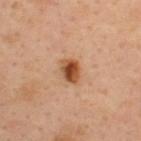<case>
<biopsy_status>not biopsied; imaged during a skin examination</biopsy_status>
<image>
  <source>total-body photography crop</source>
  <field_of_view_mm>15</field_of_view_mm>
</image>
<patient>
  <sex>male</sex>
  <age_approx>35</age_approx>
</patient>
<lesion_size>
  <long_diameter_mm_approx>2.5</long_diameter_mm_approx>
</lesion_size>
<site>upper back</site>
</case>